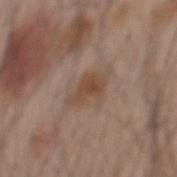Imaged during a routine full-body skin examination; the lesion was not biopsied and no histopathology is available.
Longest diameter approximately 3.5 mm.
Imaged with white-light lighting.
The subject is a male about 60 years old.
A 15 mm close-up extracted from a 3D total-body photography capture.
From the mid back.
An algorithmic analysis of the crop reported a footprint of about 5.5 mm² and a shape eccentricity near 0.8. The analysis additionally found roughly 8 lightness units darker than nearby skin and a normalized lesion–skin contrast near 7. The software also gave a classifier nevus-likeness of about 35/100 and a detector confidence of about 100 out of 100 that the crop contains a lesion.